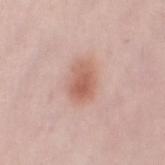| field | value |
|---|---|
| workup | total-body-photography surveillance lesion; no biopsy |
| location | the left thigh |
| patient | female, aged around 30 |
| imaging modality | 15 mm crop, total-body photography |
| tile lighting | white-light illumination |